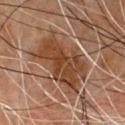notes: imaged on a skin check; not biopsied | subject: male, aged around 50 | size: ~7.5 mm (longest diameter) | image: total-body-photography crop, ~15 mm field of view | location: the chest.